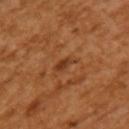workup — imaged on a skin check; not biopsied | imaging modality — ~15 mm crop, total-body skin-cancer survey | lesion diameter — ~3 mm (longest diameter) | body site — the left upper arm | tile lighting — cross-polarized | patient — female, approximately 55 years of age.